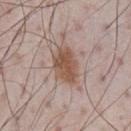Case summary:
• follow-up · total-body-photography surveillance lesion; no biopsy
• subject · male, about 70 years old
• site · the chest
• size · about 4.5 mm
• imaging modality · ~15 mm crop, total-body skin-cancer survey